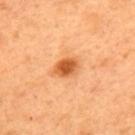Q: Illumination type?
A: cross-polarized illumination
Q: What are the patient's age and sex?
A: male, aged 48 to 52
Q: What kind of image is this?
A: ~15 mm tile from a whole-body skin photo
Q: How large is the lesion?
A: ≈3.5 mm
Q: What is the anatomic site?
A: the back